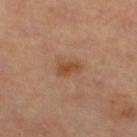Context: The patient is a female aged 68–72. The lesion is located on the left lower leg. Automated tile analysis of the lesion measured a lesion color around L≈49 a*≈22 b*≈34 in CIELAB, roughly 9 lightness units darker than nearby skin, and a normalized lesion–skin contrast near 7.5. The analysis additionally found a nevus-likeness score of about 60/100 and a lesion-detection confidence of about 100/100. Measured at roughly 2.5 mm in maximum diameter. Cropped from a total-body skin-imaging series; the visible field is about 15 mm. Imaged with cross-polarized lighting.